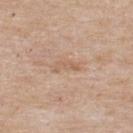notes: catalogued during a skin exam; not biopsied | automated metrics: an area of roughly 3.5 mm², a shape eccentricity near 0.9, and a shape-asymmetry score of about 0.35 (0 = symmetric); an average lesion color of about L≈60 a*≈18 b*≈31 (CIELAB), about 7 CIELAB-L* units darker than the surrounding skin, and a normalized border contrast of about 5; a within-lesion color-variation index near 0/10 and a peripheral color-asymmetry measure near 0; an automated nevus-likeness rating near 0 out of 100 and a lesion-detection confidence of about 100/100 | anatomic site: the upper back | lesion diameter: ~3 mm (longest diameter) | image source: 15 mm crop, total-body photography | patient: male, roughly 55 years of age | tile lighting: white-light illumination.